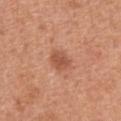workup — imaged on a skin check; not biopsied
image — total-body-photography crop, ~15 mm field of view
illumination — white-light illumination
body site — the front of the torso
patient — male, in their mid-60s
diameter — ≈3 mm